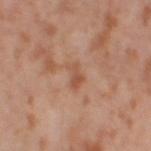The lesion was photographed on a routine skin check and not biopsied; there is no pathology result. The patient is a female aged around 55. From the left thigh. Automated tile analysis of the lesion measured a mean CIELAB color near L≈53 a*≈23 b*≈32, roughly 7 lightness units darker than nearby skin, and a lesion-to-skin contrast of about 6 (normalized; higher = more distinct). And it measured a border-irregularity rating of about 5/10 and internal color variation of about 1 on a 0–10 scale. Imaged with cross-polarized lighting. A region of skin cropped from a whole-body photographic capture, roughly 15 mm wide.